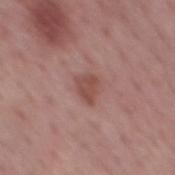Recorded during total-body skin imaging; not selected for excision or biopsy.
From the mid back.
This image is a 15 mm lesion crop taken from a total-body photograph.
The lesion-visualizer software estimated an average lesion color of about L≈48 a*≈23 b*≈24 (CIELAB), about 9 CIELAB-L* units darker than the surrounding skin, and a normalized border contrast of about 7.
The patient is a male about 75 years old.